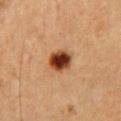Imaged during a routine full-body skin examination; the lesion was not biopsied and no histopathology is available. A lesion tile, about 15 mm wide, cut from a 3D total-body photograph. Captured under cross-polarized illumination. A male subject, roughly 50 years of age. About 3.5 mm across. On the chest.